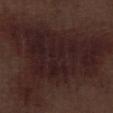* follow-up: total-body-photography surveillance lesion; no biopsy
* site: the right lower leg
* subject: male, aged approximately 70
* lighting: white-light illumination
* image source: ~15 mm tile from a whole-body skin photo